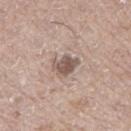Notes:
* workup — imaged on a skin check; not biopsied
* imaging modality — ~15 mm tile from a whole-body skin photo
* illumination — white-light
* site — the left thigh
* lesion size — ~3 mm (longest diameter)
* subject — male, approximately 65 years of age